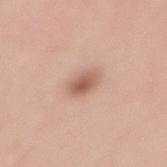Clinical impression: The lesion was tiled from a total-body skin photograph and was not biopsied. Image and clinical context: The lesion is located on the lower back. The total-body-photography lesion software estimated a shape eccentricity near 0.65 and a symmetry-axis asymmetry near 0.25. The software also gave a mean CIELAB color near L≈59 a*≈21 b*≈28 and about 12 CIELAB-L* units darker than the surrounding skin. The software also gave border irregularity of about 2 on a 0–10 scale and a within-lesion color-variation index near 3.5/10. Measured at roughly 3 mm in maximum diameter. A roughly 15 mm field-of-view crop from a total-body skin photograph. The subject is a male in their mid- to late 60s. The tile uses white-light illumination.